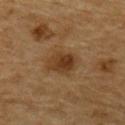workup: total-body-photography surveillance lesion; no biopsy